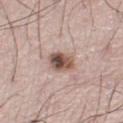The lesion was tiled from a total-body skin photograph and was not biopsied.
A male patient, aged approximately 70.
Located on the right leg.
This image is a 15 mm lesion crop taken from a total-body photograph.